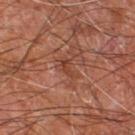Case summary:
• notes · imaged on a skin check; not biopsied
• tile lighting · cross-polarized illumination
• TBP lesion metrics · a lesion area of about 2.5 mm² and two-axis asymmetry of about 0.4
• body site · the leg
• lesion size · ≈2.5 mm
• subject · male, approximately 60 years of age
• acquisition · ~15 mm tile from a whole-body skin photo